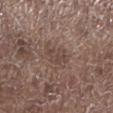Q: Was a biopsy performed?
A: imaged on a skin check; not biopsied
Q: What lighting was used for the tile?
A: white-light
Q: How large is the lesion?
A: ≈3.5 mm
Q: Who is the patient?
A: male, aged approximately 70
Q: What is the imaging modality?
A: total-body-photography crop, ~15 mm field of view
Q: Lesion location?
A: the left lower leg